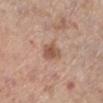biopsy status: total-body-photography surveillance lesion; no biopsy | site: the left lower leg | subject: female, roughly 65 years of age | imaging modality: ~15 mm crop, total-body skin-cancer survey.